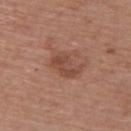site = the back
patient = female, aged 58 to 62
image source = 15 mm crop, total-body photography
lesion size = ~4 mm (longest diameter)
lighting = white-light